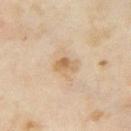Assessment: This lesion was catalogued during total-body skin photography and was not selected for biopsy. Image and clinical context: A female patient about 35 years old. About 2.5 mm across. The total-body-photography lesion software estimated an area of roughly 4 mm² and an outline eccentricity of about 0.7 (0 = round, 1 = elongated). And it measured a mean CIELAB color near L≈61 a*≈15 b*≈35, about 9 CIELAB-L* units darker than the surrounding skin, and a normalized lesion–skin contrast near 7. It also reported border irregularity of about 3 on a 0–10 scale, internal color variation of about 5 on a 0–10 scale, and a peripheral color-asymmetry measure near 1.5. The lesion is on the left upper arm. Captured under cross-polarized illumination. A lesion tile, about 15 mm wide, cut from a 3D total-body photograph.